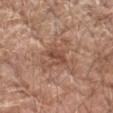Impression: Recorded during total-body skin imaging; not selected for excision or biopsy. Background: A male patient approximately 60 years of age. A lesion tile, about 15 mm wide, cut from a 3D total-body photograph. Captured under white-light illumination. The lesion-visualizer software estimated an area of roughly 5 mm², an outline eccentricity of about 0.8 (0 = round, 1 = elongated), and two-axis asymmetry of about 0.25. And it measured a lesion–skin lightness drop of about 8 and a normalized border contrast of about 6.5. The software also gave a border-irregularity rating of about 2.5/10, a color-variation rating of about 2.5/10, and radial color variation of about 1. The analysis additionally found a nevus-likeness score of about 0/100. Approximately 3 mm at its widest. On the left forearm.